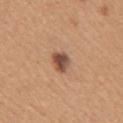{
  "biopsy_status": "not biopsied; imaged during a skin examination",
  "image": {
    "source": "total-body photography crop",
    "field_of_view_mm": 15
  },
  "patient": {
    "sex": "female",
    "age_approx": 30
  },
  "automated_metrics": {
    "area_mm2_approx": 5.0,
    "eccentricity": 0.45,
    "cielab_L": 50,
    "cielab_a": 21,
    "cielab_b": 29,
    "vs_skin_darker_L": 14.0,
    "vs_skin_contrast_norm": 10.5,
    "border_irregularity_0_10": 2.0,
    "color_variation_0_10": 6.0,
    "nevus_likeness_0_100": 85,
    "lesion_detection_confidence_0_100": 100
  },
  "site": "right upper arm",
  "lesion_size": {
    "long_diameter_mm_approx": 2.5
  }
}